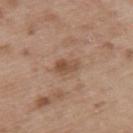Q: Is there a histopathology result?
A: no biopsy performed (imaged during a skin exam)
Q: How was the tile lit?
A: white-light
Q: What is the lesion's diameter?
A: ~3 mm (longest diameter)
Q: What are the patient's age and sex?
A: male, aged 48–52
Q: What is the imaging modality?
A: total-body-photography crop, ~15 mm field of view
Q: Automated lesion metrics?
A: a mean CIELAB color near L≈50 a*≈19 b*≈30, a lesion–skin lightness drop of about 9, and a lesion-to-skin contrast of about 7 (normalized; higher = more distinct); internal color variation of about 3 on a 0–10 scale and a peripheral color-asymmetry measure near 1.5; a classifier nevus-likeness of about 0/100 and a detector confidence of about 100 out of 100 that the crop contains a lesion
Q: Lesion location?
A: the upper back